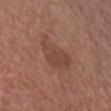Recorded during total-body skin imaging; not selected for excision or biopsy.
From the chest.
A 15 mm crop from a total-body photograph taken for skin-cancer surveillance.
Longest diameter approximately 5.5 mm.
The total-body-photography lesion software estimated an eccentricity of roughly 0.9. The analysis additionally found a mean CIELAB color near L≈44 a*≈21 b*≈27, roughly 7 lightness units darker than nearby skin, and a normalized border contrast of about 5.5. And it measured lesion-presence confidence of about 100/100.
Captured under white-light illumination.
A male subject, in their mid-60s.